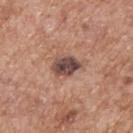follow-up: no biopsy performed (imaged during a skin exam) | subject: male, about 60 years old | diameter: about 3.5 mm | image-analysis metrics: a lesion area of about 6.5 mm² and an eccentricity of roughly 0.6; a mean CIELAB color near L≈46 a*≈19 b*≈22, a lesion–skin lightness drop of about 16, and a lesion-to-skin contrast of about 12 (normalized; higher = more distinct); border irregularity of about 2.5 on a 0–10 scale, internal color variation of about 5 on a 0–10 scale, and peripheral color asymmetry of about 2; a nevus-likeness score of about 25/100 and a lesion-detection confidence of about 100/100 | anatomic site: the back | imaging modality: 15 mm crop, total-body photography | lighting: white-light illumination.